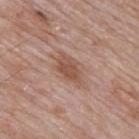notes: catalogued during a skin exam; not biopsied | TBP lesion metrics: a lesion area of about 8 mm², an eccentricity of roughly 0.8, and a shape-asymmetry score of about 0.3 (0 = symmetric); an automated nevus-likeness rating near 80 out of 100 and a lesion-detection confidence of about 100/100 | location: the mid back | subject: male, about 60 years old | diameter: ≈4.5 mm | illumination: white-light | imaging modality: 15 mm crop, total-body photography.